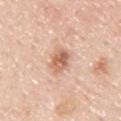Recorded during total-body skin imaging; not selected for excision or biopsy. The lesion is on the upper back. A male patient roughly 45 years of age. The total-body-photography lesion software estimated a lesion area of about 7 mm² and an outline eccentricity of about 0.4 (0 = round, 1 = elongated). The software also gave an average lesion color of about L≈64 a*≈21 b*≈32 (CIELAB), a lesion–skin lightness drop of about 12, and a normalized lesion–skin contrast near 7.5. It also reported a border-irregularity index near 2/10. A 15 mm crop from a total-body photograph taken for skin-cancer surveillance. Imaged with white-light lighting. The lesion's longest dimension is about 3 mm.